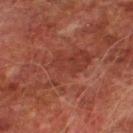A roughly 15 mm field-of-view crop from a total-body skin photograph. Automated image analysis of the tile measured a mean CIELAB color near L≈31 a*≈24 b*≈25, about 5 CIELAB-L* units darker than the surrounding skin, and a normalized lesion–skin contrast near 5. The analysis additionally found a classifier nevus-likeness of about 0/100. A male subject, aged 73 to 77. The lesion is on the left lower leg.